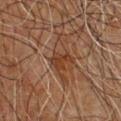Impression:
This lesion was catalogued during total-body skin photography and was not selected for biopsy.
Image and clinical context:
Longest diameter approximately 3 mm. Cropped from a whole-body photographic skin survey; the tile spans about 15 mm. This is a cross-polarized tile. A male subject aged 58 to 62. From the chest.